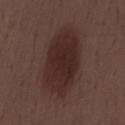Clinical impression:
This lesion was catalogued during total-body skin photography and was not selected for biopsy.
Acquisition and patient details:
The tile uses white-light illumination. Automated image analysis of the tile measured a lesion color around L≈26 a*≈18 b*≈17 in CIELAB, roughly 9 lightness units darker than nearby skin, and a normalized lesion–skin contrast near 9.5. A male patient aged approximately 50. A roughly 15 mm field-of-view crop from a total-body skin photograph. Longest diameter approximately 8.5 mm.